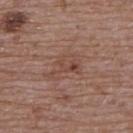Notes:
- notes · no biopsy performed (imaged during a skin exam)
- lighting · white-light illumination
- lesion diameter · ≈4 mm
- imaging modality · total-body-photography crop, ~15 mm field of view
- anatomic site · the upper back
- subject · female, in their mid- to late 60s